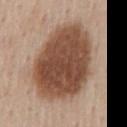notes: total-body-photography surveillance lesion; no biopsy | image source: total-body-photography crop, ~15 mm field of view | site: the mid back | patient: male, aged around 60.